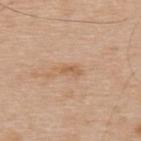Impression:
Part of a total-body skin-imaging series; this lesion was reviewed on a skin check and was not flagged for biopsy.
Context:
The lesion is located on the upper back. The subject is a male approximately 70 years of age. A region of skin cropped from a whole-body photographic capture, roughly 15 mm wide. Captured under white-light illumination. About 2.5 mm across.